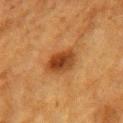Q: Was this lesion biopsied?
A: catalogued during a skin exam; not biopsied
Q: What is the imaging modality?
A: ~15 mm tile from a whole-body skin photo
Q: What lighting was used for the tile?
A: cross-polarized
Q: What is the anatomic site?
A: the right forearm
Q: Patient demographics?
A: female, in their mid- to late 50s
Q: What is the lesion's diameter?
A: about 4 mm
Q: Automated lesion metrics?
A: a footprint of about 9 mm², an outline eccentricity of about 0.75 (0 = round, 1 = elongated), and a shape-asymmetry score of about 0.15 (0 = symmetric); an average lesion color of about L≈36 a*≈23 b*≈35 (CIELAB), a lesion–skin lightness drop of about 11, and a normalized border contrast of about 9.5; radial color variation of about 2.5